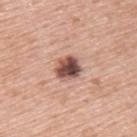Assessment:
Recorded during total-body skin imaging; not selected for excision or biopsy.
Clinical summary:
A male subject aged around 60. Located on the upper back. The tile uses white-light illumination. Measured at roughly 3 mm in maximum diameter. A roughly 15 mm field-of-view crop from a total-body skin photograph.